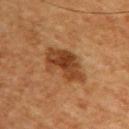Recorded during total-body skin imaging; not selected for excision or biopsy. The lesion's longest dimension is about 6 mm. A male subject aged 58 to 62. The lesion is on the chest. A roughly 15 mm field-of-view crop from a total-body skin photograph. This is a cross-polarized tile.